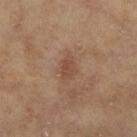The recorded lesion diameter is about 3 mm. On the left lower leg. The tile uses cross-polarized illumination. The total-body-photography lesion software estimated an outline eccentricity of about 0.75 (0 = round, 1 = elongated) and a symmetry-axis asymmetry near 0.3. The software also gave a lesion color around L≈47 a*≈18 b*≈29 in CIELAB, about 7 CIELAB-L* units darker than the surrounding skin, and a lesion-to-skin contrast of about 6 (normalized; higher = more distinct). It also reported border irregularity of about 3 on a 0–10 scale. A female subject in their mid-70s. A close-up tile cropped from a whole-body skin photograph, about 15 mm across.